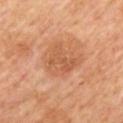Imaged during a routine full-body skin examination; the lesion was not biopsied and no histopathology is available.
The patient is aged 63–67.
From the back.
A lesion tile, about 15 mm wide, cut from a 3D total-body photograph.
About 4 mm across.
An algorithmic analysis of the crop reported a footprint of about 11 mm², an outline eccentricity of about 0.7 (0 = round, 1 = elongated), and a shape-asymmetry score of about 0.45 (0 = symmetric). It also reported an average lesion color of about L≈56 a*≈24 b*≈36 (CIELAB) and a normalized border contrast of about 5.5. The analysis additionally found a detector confidence of about 100 out of 100 that the crop contains a lesion.